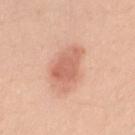The lesion was tiled from a total-body skin photograph and was not biopsied. This is a white-light tile. A female subject aged approximately 25. This image is a 15 mm lesion crop taken from a total-body photograph. The recorded lesion diameter is about 4.5 mm. The lesion is on the right upper arm.